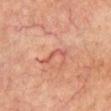follow-up: total-body-photography surveillance lesion; no biopsy | tile lighting: cross-polarized illumination | patient: male, aged 73–77 | location: the chest | image source: total-body-photography crop, ~15 mm field of view | lesion size: ~3.5 mm (longest diameter).